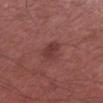Case summary:
• imaging modality: 15 mm crop, total-body photography
• subject: male, about 55 years old
• lesion size: about 3 mm
• anatomic site: the right upper arm